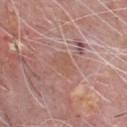biopsy status: imaged on a skin check; not biopsied
site: the chest
lesion diameter: about 3 mm
tile lighting: white-light
image: ~15 mm tile from a whole-body skin photo
subject: male, aged 58 to 62